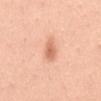biopsy status: total-body-photography surveillance lesion; no biopsy | anatomic site: the mid back | lighting: white-light | automated metrics: a footprint of about 4 mm², an outline eccentricity of about 0.9 (0 = round, 1 = elongated), and a symmetry-axis asymmetry near 0.2; a lesion–skin lightness drop of about 12 and a normalized lesion–skin contrast near 7; a border-irregularity index near 2.5/10 and peripheral color asymmetry of about 0.5; a nevus-likeness score of about 95/100 and a lesion-detection confidence of about 100/100 | size: ~3.5 mm (longest diameter) | patient: female, aged approximately 35 | image source: ~15 mm crop, total-body skin-cancer survey.